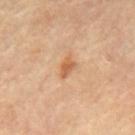<record>
<site>mid back</site>
<lesion_size>
  <long_diameter_mm_approx>2.5</long_diameter_mm_approx>
</lesion_size>
<image>
  <source>total-body photography crop</source>
  <field_of_view_mm>15</field_of_view_mm>
</image>
<lighting>cross-polarized</lighting>
<patient>
  <sex>male</sex>
  <age_approx>70</age_approx>
</patient>
</record>